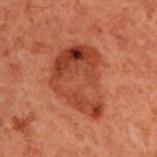Assessment:
Part of a total-body skin-imaging series; this lesion was reviewed on a skin check and was not flagged for biopsy.
Background:
A male subject, in their 60s. This is a cross-polarized tile. Automated image analysis of the tile measured a border-irregularity rating of about 3.5/10 and a color-variation rating of about 6.5/10. The software also gave an automated nevus-likeness rating near 0 out of 100 and a lesion-detection confidence of about 100/100. Located on the upper back. Measured at roughly 7.5 mm in maximum diameter. This image is a 15 mm lesion crop taken from a total-body photograph.